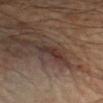Q: Is there a histopathology result?
A: catalogued during a skin exam; not biopsied
Q: Patient demographics?
A: male, in their 70s
Q: How was this image acquired?
A: ~15 mm crop, total-body skin-cancer survey
Q: What is the anatomic site?
A: the left upper arm